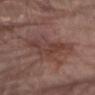{
  "image": {
    "source": "total-body photography crop",
    "field_of_view_mm": 15
  },
  "site": "arm",
  "lighting": "white-light",
  "lesion_size": {
    "long_diameter_mm_approx": 7.5
  },
  "patient": {
    "sex": "male",
    "age_approx": 80
  }
}